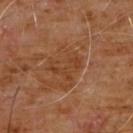Impression: The lesion was photographed on a routine skin check and not biopsied; there is no pathology result. Image and clinical context: A male patient aged around 60. The recorded lesion diameter is about 4 mm. On the chest. A 15 mm crop from a total-body photograph taken for skin-cancer surveillance.